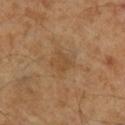Impression: Recorded during total-body skin imaging; not selected for excision or biopsy. Acquisition and patient details: A male subject, about 65 years old. The lesion-visualizer software estimated internal color variation of about 1.5 on a 0–10 scale and a peripheral color-asymmetry measure near 0.5. A roughly 15 mm field-of-view crop from a total-body skin photograph.